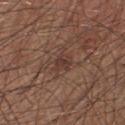Q: Was this lesion biopsied?
A: imaged on a skin check; not biopsied
Q: How was the tile lit?
A: white-light illumination
Q: Patient demographics?
A: male, in their 60s
Q: What is the imaging modality?
A: total-body-photography crop, ~15 mm field of view
Q: What is the anatomic site?
A: the right lower leg